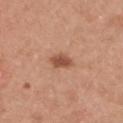<tbp_lesion>
<biopsy_status>not biopsied; imaged during a skin examination</biopsy_status>
</tbp_lesion>